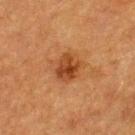Impression:
The lesion was photographed on a routine skin check and not biopsied; there is no pathology result.
Acquisition and patient details:
The subject is a female approximately 55 years of age. The lesion's longest dimension is about 3.5 mm. The lesion is located on the upper back. An algorithmic analysis of the crop reported a mean CIELAB color near L≈37 a*≈23 b*≈34, roughly 10 lightness units darker than nearby skin, and a normalized border contrast of about 8.5. The analysis additionally found a border-irregularity rating of about 2.5/10, a color-variation rating of about 4.5/10, and a peripheral color-asymmetry measure near 2. And it measured a classifier nevus-likeness of about 95/100 and lesion-presence confidence of about 100/100. The tile uses cross-polarized illumination. This image is a 15 mm lesion crop taken from a total-body photograph.